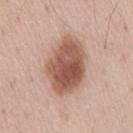biopsy status: total-body-photography surveillance lesion; no biopsy | location: the back | image source: 15 mm crop, total-body photography | image-analysis metrics: a footprint of about 24 mm², an outline eccentricity of about 0.75 (0 = round, 1 = elongated), and a shape-asymmetry score of about 0.15 (0 = symmetric); about 15 CIELAB-L* units darker than the surrounding skin and a normalized lesion–skin contrast near 10; border irregularity of about 2 on a 0–10 scale, a within-lesion color-variation index near 5.5/10, and radial color variation of about 2; a detector confidence of about 100 out of 100 that the crop contains a lesion | lighting: white-light | diameter: about 7 mm | subject: male, aged 53–57.